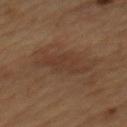image source: ~15 mm tile from a whole-body skin photo | image-analysis metrics: lesion-presence confidence of about 100/100 | body site: the mid back | lighting: cross-polarized illumination | subject: male, about 55 years old | lesion size: about 6 mm.